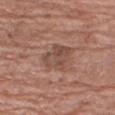<tbp_lesion>
  <site>right upper arm</site>
  <lighting>white-light</lighting>
  <lesion_size>
    <long_diameter_mm_approx>4.0</long_diameter_mm_approx>
  </lesion_size>
  <patient>
    <sex>male</sex>
    <age_approx>75</age_approx>
  </patient>
  <image>
    <source>total-body photography crop</source>
    <field_of_view_mm>15</field_of_view_mm>
  </image>
</tbp_lesion>